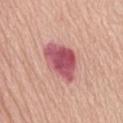workup: total-body-photography surveillance lesion; no biopsy | image source: 15 mm crop, total-body photography | patient: female, aged approximately 75 | lighting: white-light | site: the abdomen | automated metrics: a footprint of about 14 mm², an outline eccentricity of about 0.7 (0 = round, 1 = elongated), and a symmetry-axis asymmetry near 0.2; an automated nevus-likeness rating near 5 out of 100 | size: ≈5 mm.